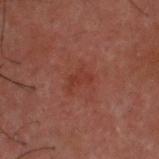Notes:
– notes: imaged on a skin check; not biopsied
– lesion size: ≈3 mm
– illumination: cross-polarized
– automated metrics: an area of roughly 4 mm² and a shape-asymmetry score of about 0.4 (0 = symmetric); an average lesion color of about L≈26 a*≈21 b*≈21 (CIELAB), roughly 4 lightness units darker than nearby skin, and a normalized border contrast of about 5; internal color variation of about 1.5 on a 0–10 scale and a peripheral color-asymmetry measure near 0.5
– image source: ~15 mm crop, total-body skin-cancer survey
– patient: male, aged approximately 50
– body site: the head or neck